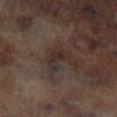| key | value |
|---|---|
| notes | total-body-photography surveillance lesion; no biopsy |
| image source | total-body-photography crop, ~15 mm field of view |
| site | the leg |
| tile lighting | cross-polarized |
| diameter | ≈3.5 mm |
| automated lesion analysis | a within-lesion color-variation index near 2.5/10 and a peripheral color-asymmetry measure near 1 |
| patient | male, aged 63–67 |